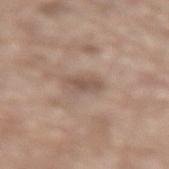  image:
    source: total-body photography crop
    field_of_view_mm: 15
  patient:
    sex: male
    age_approx: 80
  lighting: white-light
  site: lower back